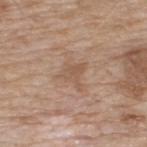{
  "biopsy_status": "not biopsied; imaged during a skin examination",
  "site": "upper back",
  "patient": {
    "sex": "male",
    "age_approx": 65
  },
  "lighting": "white-light",
  "image": {
    "source": "total-body photography crop",
    "field_of_view_mm": 15
  }
}